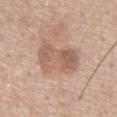image: total-body-photography crop, ~15 mm field of view; patient: male, aged 58–62; body site: the chest.